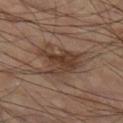The subject is a male aged approximately 60.
Longest diameter approximately 5.5 mm.
This image is a 15 mm lesion crop taken from a total-body photograph.
An algorithmic analysis of the crop reported a footprint of about 13 mm², a shape eccentricity near 0.7, and two-axis asymmetry of about 0.45. The software also gave a lesion color around L≈37 a*≈16 b*≈25 in CIELAB, about 9 CIELAB-L* units darker than the surrounding skin, and a lesion-to-skin contrast of about 8 (normalized; higher = more distinct). It also reported a border-irregularity index near 5.5/10, internal color variation of about 4 on a 0–10 scale, and peripheral color asymmetry of about 1.5. It also reported lesion-presence confidence of about 100/100.
The lesion is located on the left thigh.
Captured under cross-polarized illumination.